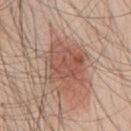Impression:
This lesion was catalogued during total-body skin photography and was not selected for biopsy.
Context:
The lesion is on the chest. A male subject, aged 43 to 47. Automated image analysis of the tile measured a lesion area of about 21 mm² and an outline eccentricity of about 0.5 (0 = round, 1 = elongated). It also reported a lesion–skin lightness drop of about 10 and a lesion-to-skin contrast of about 7 (normalized; higher = more distinct). A lesion tile, about 15 mm wide, cut from a 3D total-body photograph. The recorded lesion diameter is about 6 mm.